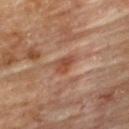{"biopsy_status": "not biopsied; imaged during a skin examination", "lesion_size": {"long_diameter_mm_approx": 2.5}, "image": {"source": "total-body photography crop", "field_of_view_mm": 15}, "automated_metrics": {"area_mm2_approx": 3.5, "eccentricity": 0.75, "shape_asymmetry": 0.3, "border_irregularity_0_10": 2.5, "color_variation_0_10": 2.0, "peripheral_color_asymmetry": 0.5, "nevus_likeness_0_100": 20, "lesion_detection_confidence_0_100": 100}, "lighting": "cross-polarized", "patient": {"sex": "male", "age_approx": "85+"}, "site": "upper back"}